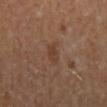biopsy status = no biopsy performed (imaged during a skin exam)
image = ~15 mm tile from a whole-body skin photo
site = the back
illumination = cross-polarized illumination
diameter = about 3 mm
subject = male, in their mid- to late 60s